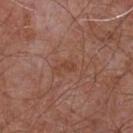Findings:
* notes — total-body-photography surveillance lesion; no biopsy
* acquisition — ~15 mm tile from a whole-body skin photo
* location — the chest
* subject — male, aged 53 to 57